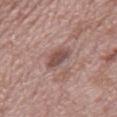Impression: This lesion was catalogued during total-body skin photography and was not selected for biopsy. Background: The recorded lesion diameter is about 3 mm. A 15 mm crop from a total-body photograph taken for skin-cancer surveillance. The lesion is on the right thigh. The patient is a male approximately 55 years of age. Imaged with white-light lighting. An algorithmic analysis of the crop reported an outline eccentricity of about 0.75 (0 = round, 1 = elongated) and two-axis asymmetry of about 0.3. The software also gave a lesion–skin lightness drop of about 11 and a normalized border contrast of about 7.5. And it measured a classifier nevus-likeness of about 0/100 and a lesion-detection confidence of about 100/100.